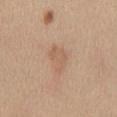Part of a total-body skin-imaging series; this lesion was reviewed on a skin check and was not flagged for biopsy.
Automated image analysis of the tile measured a border-irregularity index near 4/10, a color-variation rating of about 2/10, and peripheral color asymmetry of about 1.
The lesion is located on the mid back.
This image is a 15 mm lesion crop taken from a total-body photograph.
About 3.5 mm across.
The subject is a female aged 38 to 42.
The tile uses white-light illumination.